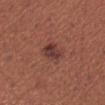Recorded during total-body skin imaging; not selected for excision or biopsy.
Imaged with white-light lighting.
A male patient, roughly 45 years of age.
From the arm.
Measured at roughly 3.5 mm in maximum diameter.
A roughly 15 mm field-of-view crop from a total-body skin photograph.